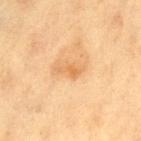| feature | finding |
|---|---|
| follow-up | no biopsy performed (imaged during a skin exam) |
| patient | female, aged approximately 40 |
| imaging modality | ~15 mm tile from a whole-body skin photo |
| site | the left thigh |
| size | ~3 mm (longest diameter) |
| lighting | cross-polarized illumination |